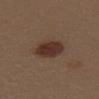Q: What lighting was used for the tile?
A: white-light illumination
Q: What are the patient's age and sex?
A: female, roughly 40 years of age
Q: What is the imaging modality?
A: 15 mm crop, total-body photography
Q: Automated lesion metrics?
A: a mean CIELAB color near L≈32 a*≈18 b*≈23 and a lesion-to-skin contrast of about 10 (normalized; higher = more distinct); a border-irregularity rating of about 1.5/10 and a within-lesion color-variation index near 2.5/10
Q: What is the anatomic site?
A: the back
Q: What is the lesion's diameter?
A: ≈3.5 mm